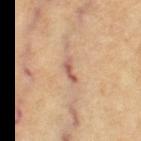Impression:
The lesion was photographed on a routine skin check and not biopsied; there is no pathology result.
Image and clinical context:
From the left thigh. The patient is a female in their mid-60s. A lesion tile, about 15 mm wide, cut from a 3D total-body photograph.